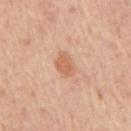Q: Was this lesion biopsied?
A: no biopsy performed (imaged during a skin exam)
Q: How large is the lesion?
A: ~3 mm (longest diameter)
Q: What is the anatomic site?
A: the right upper arm
Q: Patient demographics?
A: male, roughly 75 years of age
Q: How was the tile lit?
A: white-light
Q: What kind of image is this?
A: ~15 mm tile from a whole-body skin photo